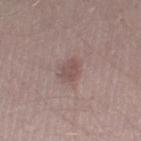Recorded during total-body skin imaging; not selected for excision or biopsy. Longest diameter approximately 3 mm. An algorithmic analysis of the crop reported an average lesion color of about L≈50 a*≈18 b*≈19 (CIELAB) and roughly 8 lightness units darker than nearby skin. The software also gave a border-irregularity index near 2.5/10, a color-variation rating of about 2.5/10, and radial color variation of about 1. A roughly 15 mm field-of-view crop from a total-body skin photograph. A male subject, aged 28 to 32. Captured under white-light illumination.